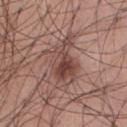The lesion was tiled from a total-body skin photograph and was not biopsied. The lesion-visualizer software estimated a lesion area of about 18 mm² and an eccentricity of roughly 0.8. The software also gave a mean CIELAB color near L≈47 a*≈20 b*≈23, about 10 CIELAB-L* units darker than the surrounding skin, and a normalized border contrast of about 7.5. This image is a 15 mm lesion crop taken from a total-body photograph. A male patient in their 30s. Located on the chest. This is a white-light tile. The recorded lesion diameter is about 6.5 mm.